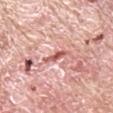* biopsy status: imaged on a skin check; not biopsied
* diameter: ≈2.5 mm
* automated lesion analysis: a shape eccentricity near 0.9 and a shape-asymmetry score of about 0.35 (0 = symmetric); an average lesion color of about L≈58 a*≈30 b*≈27 (CIELAB) and a normalized lesion–skin contrast near 8.5; a border-irregularity index near 4.5/10 and a within-lesion color-variation index near 0/10
* patient: male, aged 63–67
* image: ~15 mm crop, total-body skin-cancer survey
* site: the right upper arm
* lighting: white-light